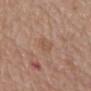This lesion was catalogued during total-body skin photography and was not selected for biopsy. The lesion's longest dimension is about 2.5 mm. Imaged with white-light lighting. Cropped from a whole-body photographic skin survey; the tile spans about 15 mm. A male subject aged around 80. The total-body-photography lesion software estimated an outline eccentricity of about 0.8 (0 = round, 1 = elongated) and two-axis asymmetry of about 0.35. Located on the mid back.